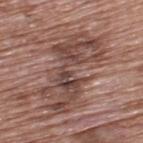follow-up = no biopsy performed (imaged during a skin exam).